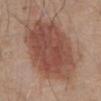<tbp_lesion>
  <biopsy_status>not biopsied; imaged during a skin examination</biopsy_status>
  <lesion_size>
    <long_diameter_mm_approx>9.0</long_diameter_mm_approx>
  </lesion_size>
  <site>abdomen</site>
  <image>
    <source>total-body photography crop</source>
    <field_of_view_mm>15</field_of_view_mm>
  </image>
  <patient>
    <sex>male</sex>
    <age_approx>80</age_approx>
  </patient>
</tbp_lesion>